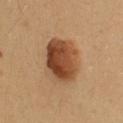No biopsy was performed on this lesion — it was imaged during a full skin examination and was not determined to be concerning. A female patient aged around 35. A 15 mm close-up tile from a total-body photography series done for melanoma screening. The lesion is on the upper back.